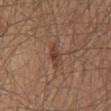Assessment:
No biopsy was performed on this lesion — it was imaged during a full skin examination and was not determined to be concerning.
Image and clinical context:
This is a white-light tile. From the abdomen. An algorithmic analysis of the crop reported a lesion area of about 4.5 mm², a shape eccentricity near 0.85, and a shape-asymmetry score of about 0.25 (0 = symmetric). The software also gave an average lesion color of about L≈42 a*≈19 b*≈27 (CIELAB) and a lesion-to-skin contrast of about 7 (normalized; higher = more distinct). It also reported a border-irregularity rating of about 3/10 and a within-lesion color-variation index near 2.5/10. The analysis additionally found a classifier nevus-likeness of about 10/100 and lesion-presence confidence of about 100/100. This image is a 15 mm lesion crop taken from a total-body photograph. The subject is a male aged around 65.